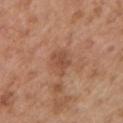Findings:
• biopsy status: no biopsy performed (imaged during a skin exam)
• subject: male, aged 53 to 57
• size: ~3 mm (longest diameter)
• automated lesion analysis: a border-irregularity rating of about 4/10, internal color variation of about 2 on a 0–10 scale, and radial color variation of about 0.5; an automated nevus-likeness rating near 0 out of 100 and a lesion-detection confidence of about 100/100
• location: the left upper arm
• imaging modality: 15 mm crop, total-body photography